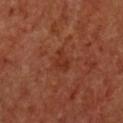Findings:
* workup · catalogued during a skin exam; not biopsied
* tile lighting · cross-polarized illumination
* image · 15 mm crop, total-body photography
* diameter · ≈2.5 mm
* body site · the chest
* image-analysis metrics · an area of roughly 4 mm², a shape eccentricity near 0.6, and two-axis asymmetry of about 0.55; a mean CIELAB color near L≈33 a*≈27 b*≈32, about 6 CIELAB-L* units darker than the surrounding skin, and a normalized border contrast of about 6; a color-variation rating of about 1.5/10 and peripheral color asymmetry of about 0.5; a classifier nevus-likeness of about 0/100
* patient · female, in their mid-60s